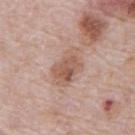biopsy status=catalogued during a skin exam; not biopsied
image source=~15 mm tile from a whole-body skin photo
diameter=≈5 mm
body site=the abdomen
patient=male, roughly 70 years of age
lighting=white-light illumination